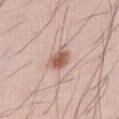Case summary:
- notes · total-body-photography surveillance lesion; no biopsy
- diameter · ≈3 mm
- site · the right thigh
- imaging modality · ~15 mm tile from a whole-body skin photo
- illumination · white-light illumination
- subject · male, in their 30s
- automated lesion analysis · a lesion area of about 5.5 mm², an outline eccentricity of about 0.65 (0 = round, 1 = elongated), and a shape-asymmetry score of about 0.2 (0 = symmetric); a mean CIELAB color near L≈58 a*≈21 b*≈26, roughly 13 lightness units darker than nearby skin, and a lesion-to-skin contrast of about 8.5 (normalized; higher = more distinct); a nevus-likeness score of about 90/100 and a lesion-detection confidence of about 100/100